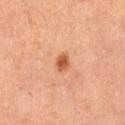Clinical impression: Recorded during total-body skin imaging; not selected for excision or biopsy. Background: A female subject aged around 55. Imaged with cross-polarized lighting. The lesion is on the leg. Automated tile analysis of the lesion measured a mean CIELAB color near L≈53 a*≈27 b*≈37, roughly 13 lightness units darker than nearby skin, and a lesion-to-skin contrast of about 9 (normalized; higher = more distinct). The software also gave a border-irregularity rating of about 1.5/10, internal color variation of about 2 on a 0–10 scale, and peripheral color asymmetry of about 0.5. Measured at roughly 2 mm in maximum diameter. This image is a 15 mm lesion crop taken from a total-body photograph.